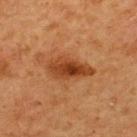Recorded during total-body skin imaging; not selected for excision or biopsy. The recorded lesion diameter is about 5.5 mm. An algorithmic analysis of the crop reported an outline eccentricity of about 0.85 (0 = round, 1 = elongated). The software also gave an average lesion color of about L≈37 a*≈24 b*≈35 (CIELAB) and a normalized border contrast of about 9. This is a cross-polarized tile. A male subject aged around 65. A region of skin cropped from a whole-body photographic capture, roughly 15 mm wide. The lesion is on the upper back.